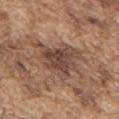Q: Was this lesion biopsied?
A: catalogued during a skin exam; not biopsied
Q: What is the lesion's diameter?
A: ≈5.5 mm
Q: Illumination type?
A: white-light illumination
Q: Lesion location?
A: the upper back
Q: How was this image acquired?
A: 15 mm crop, total-body photography
Q: Patient demographics?
A: male, in their mid-70s